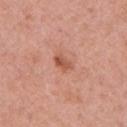Impression:
Recorded during total-body skin imaging; not selected for excision or biopsy.
Acquisition and patient details:
A close-up tile cropped from a whole-body skin photograph, about 15 mm across. The lesion's longest dimension is about 2.5 mm. On the right upper arm. A female subject, roughly 40 years of age.